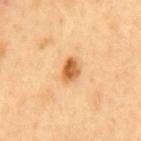Impression: Part of a total-body skin-imaging series; this lesion was reviewed on a skin check and was not flagged for biopsy. Context: The subject is a male aged approximately 50. On the back. The recorded lesion diameter is about 2.5 mm. This image is a 15 mm lesion crop taken from a total-body photograph. The tile uses cross-polarized illumination. The lesion-visualizer software estimated a mean CIELAB color near L≈62 a*≈25 b*≈45 and about 15 CIELAB-L* units darker than the surrounding skin. The analysis additionally found a peripheral color-asymmetry measure near 2. The analysis additionally found an automated nevus-likeness rating near 95 out of 100 and a detector confidence of about 100 out of 100 that the crop contains a lesion.